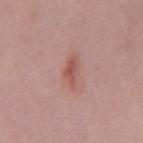biopsy status: imaged on a skin check; not biopsied
patient: male, about 50 years old
lighting: white-light illumination
diameter: ≈3.5 mm
anatomic site: the back
image-analysis metrics: an area of roughly 4 mm², an outline eccentricity of about 0.9 (0 = round, 1 = elongated), and two-axis asymmetry of about 0.45; border irregularity of about 4.5 on a 0–10 scale and internal color variation of about 2.5 on a 0–10 scale; a nevus-likeness score of about 20/100 and a detector confidence of about 100 out of 100 that the crop contains a lesion
image source: 15 mm crop, total-body photography